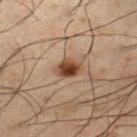Part of a total-body skin-imaging series; this lesion was reviewed on a skin check and was not flagged for biopsy.
A male patient, approximately 50 years of age.
The tile uses cross-polarized illumination.
Located on the left lower leg.
A lesion tile, about 15 mm wide, cut from a 3D total-body photograph.
The total-body-photography lesion software estimated a mean CIELAB color near L≈34 a*≈16 b*≈25, a lesion–skin lightness drop of about 12, and a lesion-to-skin contrast of about 11 (normalized; higher = more distinct). The analysis additionally found a border-irregularity index near 1.5/10, a within-lesion color-variation index near 5.5/10, and peripheral color asymmetry of about 2.
Measured at roughly 2.5 mm in maximum diameter.